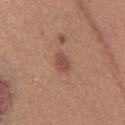Q: Was a biopsy performed?
A: no biopsy performed (imaged during a skin exam)
Q: Where on the body is the lesion?
A: the front of the torso
Q: What kind of image is this?
A: ~15 mm tile from a whole-body skin photo
Q: How was the tile lit?
A: white-light
Q: Patient demographics?
A: female, aged around 35
Q: How large is the lesion?
A: about 2.5 mm